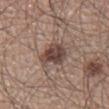Part of a total-body skin-imaging series; this lesion was reviewed on a skin check and was not flagged for biopsy. The lesion is on the right lower leg. A close-up tile cropped from a whole-body skin photograph, about 15 mm across. About 4 mm across. A male patient aged approximately 60. The total-body-photography lesion software estimated a nevus-likeness score of about 85/100 and lesion-presence confidence of about 100/100.